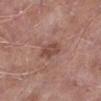Imaged during a routine full-body skin examination; the lesion was not biopsied and no histopathology is available.
The tile uses white-light illumination.
An algorithmic analysis of the crop reported an eccentricity of roughly 0.75. The analysis additionally found a classifier nevus-likeness of about 5/100 and lesion-presence confidence of about 100/100.
A male patient, aged 53–57.
Located on the left lower leg.
Measured at roughly 3 mm in maximum diameter.
A 15 mm close-up tile from a total-body photography series done for melanoma screening.